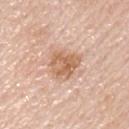Part of a total-body skin-imaging series; this lesion was reviewed on a skin check and was not flagged for biopsy.
Measured at roughly 4 mm in maximum diameter.
The lesion-visualizer software estimated a footprint of about 9.5 mm², a shape eccentricity near 0.6, and a symmetry-axis asymmetry near 0.25. The software also gave a lesion color around L≈63 a*≈21 b*≈33 in CIELAB and about 11 CIELAB-L* units darker than the surrounding skin.
Located on the right upper arm.
A close-up tile cropped from a whole-body skin photograph, about 15 mm across.
A male patient, in their 60s.
This is a white-light tile.